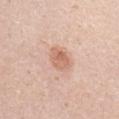Findings:
• workup · catalogued during a skin exam; not biopsied
• size · ~3 mm (longest diameter)
• location · the right upper arm
• illumination · white-light illumination
• subject · male, roughly 45 years of age
• acquisition · total-body-photography crop, ~15 mm field of view